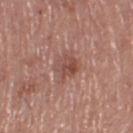Assessment:
Part of a total-body skin-imaging series; this lesion was reviewed on a skin check and was not flagged for biopsy.
Clinical summary:
Automated tile analysis of the lesion measured a footprint of about 8 mm², a shape eccentricity near 0.55, and a shape-asymmetry score of about 0.25 (0 = symmetric). The analysis additionally found a nevus-likeness score of about 0/100. Approximately 3.5 mm at its widest. A male subject in their 70s. Located on the right thigh. Imaged with white-light lighting. A 15 mm crop from a total-body photograph taken for skin-cancer surveillance.